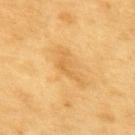biopsy status: total-body-photography surveillance lesion; no biopsy | body site: the back | patient: male, aged 58–62 | tile lighting: cross-polarized illumination | diameter: about 4.5 mm | image source: ~15 mm crop, total-body skin-cancer survey.